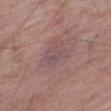Recorded during total-body skin imaging; not selected for excision or biopsy.
About 3 mm across.
The subject is a female aged 73 to 77.
On the right thigh.
Imaged with white-light lighting.
Cropped from a whole-body photographic skin survey; the tile spans about 15 mm.